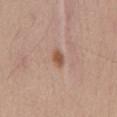Imaged during a routine full-body skin examination; the lesion was not biopsied and no histopathology is available. Cropped from a total-body skin-imaging series; the visible field is about 15 mm. A male subject, aged 58 to 62. Located on the chest.